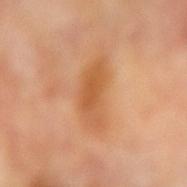Q: What is the anatomic site?
A: the right forearm
Q: How was this image acquired?
A: ~15 mm crop, total-body skin-cancer survey
Q: Who is the patient?
A: female, in their mid-70s
Q: What lighting was used for the tile?
A: cross-polarized illumination